This image is a 15 mm lesion crop taken from a total-body photograph, the patient is a male aged around 60, from the abdomen, measured at roughly 7.5 mm in maximum diameter, captured under white-light illumination.
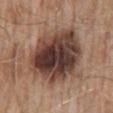Diagnosis:
The lesion was biopsied, and histopathology showed a dysplastic (Clark) nevus.